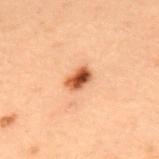This lesion was catalogued during total-body skin photography and was not selected for biopsy.
This is a cross-polarized tile.
A close-up tile cropped from a whole-body skin photograph, about 15 mm across.
The lesion-visualizer software estimated an eccentricity of roughly 0.75 and two-axis asymmetry of about 0.25. And it measured a lesion color around L≈46 a*≈24 b*≈34 in CIELAB and a normalized lesion–skin contrast near 11.5. The analysis additionally found a detector confidence of about 100 out of 100 that the crop contains a lesion.
On the upper back.
A male patient, aged 53–57.